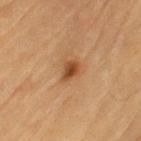Q: Lesion location?
A: the left upper arm
Q: What did automated image analysis measure?
A: an area of roughly 4 mm² and a shape-asymmetry score of about 0.3 (0 = symmetric); a border-irregularity index near 2.5/10, a within-lesion color-variation index near 4.5/10, and radial color variation of about 1.5; a nevus-likeness score of about 85/100 and a lesion-detection confidence of about 100/100
Q: What are the patient's age and sex?
A: male, aged 83 or over
Q: Illumination type?
A: cross-polarized
Q: How was this image acquired?
A: total-body-photography crop, ~15 mm field of view